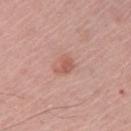{
  "biopsy_status": "not biopsied; imaged during a skin examination",
  "patient": {
    "sex": "female",
    "age_approx": 65
  },
  "lesion_size": {
    "long_diameter_mm_approx": 2.5
  },
  "lighting": "white-light",
  "site": "chest",
  "image": {
    "source": "total-body photography crop",
    "field_of_view_mm": 15
  },
  "automated_metrics": {
    "color_variation_0_10": 2.5,
    "lesion_detection_confidence_0_100": 100
  }
}